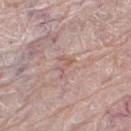{"biopsy_status": "not biopsied; imaged during a skin examination", "image": {"source": "total-body photography crop", "field_of_view_mm": 15}, "automated_metrics": {"area_mm2_approx": 2.5, "eccentricity": 0.9, "shape_asymmetry": 0.55, "cielab_L": 58, "cielab_a": 21, "cielab_b": 23, "vs_skin_darker_L": 7.0, "vs_skin_contrast_norm": 5.0, "nevus_likeness_0_100": 0, "lesion_detection_confidence_0_100": 85}, "patient": {"sex": "female", "age_approx": 65}, "site": "left thigh"}